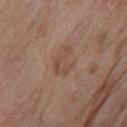Findings:
* follow-up · imaged on a skin check; not biopsied
* lighting · white-light illumination
* body site · the left upper arm
* patient · female, about 85 years old or older
* image · 15 mm crop, total-body photography
* lesion size · ~3.5 mm (longest diameter)